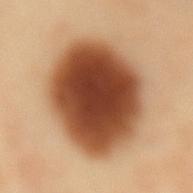{
  "biopsy_status": "not biopsied; imaged during a skin examination",
  "site": "lower back",
  "image": {
    "source": "total-body photography crop",
    "field_of_view_mm": 15
  },
  "patient": {
    "sex": "female",
    "age_approx": 50
  }
}